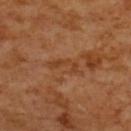biopsy status = imaged on a skin check; not biopsied | acquisition = 15 mm crop, total-body photography | body site = the upper back | lesion size = ~4.5 mm (longest diameter) | lighting = cross-polarized illumination | automated metrics = a footprint of about 4.5 mm² and an eccentricity of roughly 0.95; an average lesion color of about L≈43 a*≈24 b*≈37 (CIELAB), about 7 CIELAB-L* units darker than the surrounding skin, and a normalized border contrast of about 5.5; a detector confidence of about 50 out of 100 that the crop contains a lesion | subject = female, aged approximately 55.